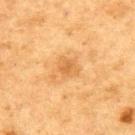Impression:
This lesion was catalogued during total-body skin photography and was not selected for biopsy.
Background:
This image is a 15 mm lesion crop taken from a total-body photograph. This is a cross-polarized tile. A male subject roughly 75 years of age. From the upper back. Approximately 2.5 mm at its widest. The total-body-photography lesion software estimated a footprint of about 4 mm², an outline eccentricity of about 0.55 (0 = round, 1 = elongated), and a symmetry-axis asymmetry near 0.35. The software also gave about 7 CIELAB-L* units darker than the surrounding skin and a normalized border contrast of about 5.5. The software also gave a border-irregularity rating of about 3/10, internal color variation of about 1.5 on a 0–10 scale, and a peripheral color-asymmetry measure near 0.5. And it measured lesion-presence confidence of about 100/100.